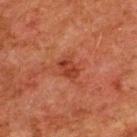This lesion was catalogued during total-body skin photography and was not selected for biopsy. An algorithmic analysis of the crop reported a within-lesion color-variation index near 3/10. It also reported a classifier nevus-likeness of about 5/100 and lesion-presence confidence of about 100/100. The lesion's longest dimension is about 2.5 mm. The lesion is on the upper back. A 15 mm crop from a total-body photograph taken for skin-cancer surveillance. The subject is a male roughly 80 years of age. The tile uses cross-polarized illumination.